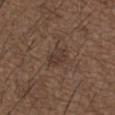Q: What is the imaging modality?
A: 15 mm crop, total-body photography
Q: Automated lesion metrics?
A: a lesion area of about 5.5 mm² and a symmetry-axis asymmetry near 0.2; an average lesion color of about L≈36 a*≈15 b*≈22 (CIELAB), about 6 CIELAB-L* units darker than the surrounding skin, and a lesion-to-skin contrast of about 6 (normalized; higher = more distinct); a border-irregularity index near 2.5/10, a color-variation rating of about 2.5/10, and radial color variation of about 0.5; an automated nevus-likeness rating near 5 out of 100 and a lesion-detection confidence of about 95/100
Q: What are the patient's age and sex?
A: male, aged 48–52
Q: How was the tile lit?
A: white-light
Q: Lesion location?
A: the back